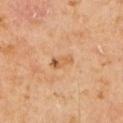follow-up = no biopsy performed (imaged during a skin exam) | TBP lesion metrics = an area of roughly 4 mm², a shape eccentricity near 0.85, and a symmetry-axis asymmetry near 0.3; a lesion color around L≈56 a*≈20 b*≈37 in CIELAB, a lesion–skin lightness drop of about 8, and a normalized border contrast of about 6; internal color variation of about 4 on a 0–10 scale and radial color variation of about 1; a nevus-likeness score of about 0/100 and lesion-presence confidence of about 100/100 | imaging modality = 15 mm crop, total-body photography | subject = male, about 60 years old | diameter = ≈3 mm | illumination = cross-polarized illumination | site = the left upper arm.